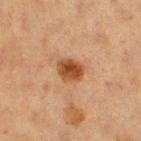notes: catalogued during a skin exam; not biopsied | subject: female, in their 40s | lesion diameter: about 3 mm | site: the right thigh | image: total-body-photography crop, ~15 mm field of view | illumination: cross-polarized.